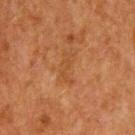No biopsy was performed on this lesion — it was imaged during a full skin examination and was not determined to be concerning. Located on the upper back. Imaged with cross-polarized lighting. A region of skin cropped from a whole-body photographic capture, roughly 15 mm wide. A male patient, approximately 50 years of age.